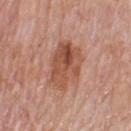• biopsy status — imaged on a skin check; not biopsied
• site — the back
• subject — male, in their mid-70s
• image source — ~15 mm tile from a whole-body skin photo